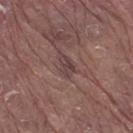biopsy status: no biopsy performed (imaged during a skin exam) | subject: male, aged approximately 80 | site: the right thigh | acquisition: 15 mm crop, total-body photography | automated metrics: an area of roughly 4 mm², a shape eccentricity near 0.8, and a shape-asymmetry score of about 0.25 (0 = symmetric); a lesion color around L≈41 a*≈18 b*≈17 in CIELAB, about 7 CIELAB-L* units darker than the surrounding skin, and a normalized border contrast of about 7 | size: ~3 mm (longest diameter) | tile lighting: white-light.